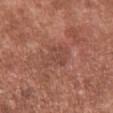biopsy_status: not biopsied; imaged during a skin examination